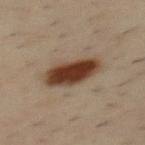Acquisition and patient details: The lesion is on the back. The subject is a male aged around 40. The lesion-visualizer software estimated a lesion area of about 15 mm², an eccentricity of roughly 0.8, and two-axis asymmetry of about 0.15. The software also gave an average lesion color of about L≈39 a*≈17 b*≈28 (CIELAB), roughly 16 lightness units darker than nearby skin, and a normalized border contrast of about 13.5. It also reported a detector confidence of about 100 out of 100 that the crop contains a lesion. A 15 mm close-up tile from a total-body photography series done for melanoma screening.